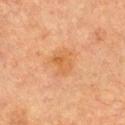The lesion was photographed on a routine skin check and not biopsied; there is no pathology result.
The total-body-photography lesion software estimated an area of roughly 6.5 mm² and an eccentricity of roughly 0.65.
The subject is a female aged 58–62.
This is a cross-polarized tile.
A close-up tile cropped from a whole-body skin photograph, about 15 mm across.
Measured at roughly 3 mm in maximum diameter.
The lesion is on the chest.